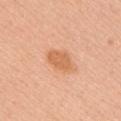{
  "biopsy_status": "not biopsied; imaged during a skin examination",
  "automated_metrics": {
    "eccentricity": 0.8,
    "cielab_L": 64,
    "cielab_a": 26,
    "cielab_b": 39,
    "vs_skin_darker_L": 9.0,
    "vs_skin_contrast_norm": 6.5,
    "border_irregularity_0_10": 2.0,
    "color_variation_0_10": 2.0,
    "lesion_detection_confidence_0_100": 100
  },
  "image": {
    "source": "total-body photography crop",
    "field_of_view_mm": 15
  },
  "lighting": "white-light",
  "patient": {
    "sex": "female",
    "age_approx": 45
  },
  "site": "chest"
}